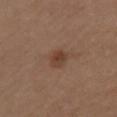Recorded during total-body skin imaging; not selected for excision or biopsy. On the left upper arm. Approximately 2.5 mm at its widest. The tile uses white-light illumination. A female patient in their 40s. A 15 mm crop from a total-body photograph taken for skin-cancer surveillance. The lesion-visualizer software estimated an average lesion color of about L≈40 a*≈19 b*≈27 (CIELAB). It also reported a nevus-likeness score of about 65/100 and a lesion-detection confidence of about 100/100.